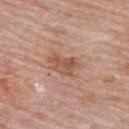Imaged during a routine full-body skin examination; the lesion was not biopsied and no histopathology is available. Measured at roughly 4.5 mm in maximum diameter. Cropped from a whole-body photographic skin survey; the tile spans about 15 mm. A female patient, roughly 65 years of age. On the back. The lesion-visualizer software estimated a nevus-likeness score of about 10/100 and lesion-presence confidence of about 100/100. Captured under white-light illumination.